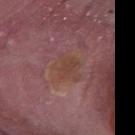Imaged with white-light lighting. The subject is a male aged approximately 40. About 3.5 mm across. The lesion is on the left lower leg. A roughly 15 mm field-of-view crop from a total-body skin photograph.